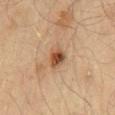  biopsy_status: not biopsied; imaged during a skin examination
  automated_metrics:
    border_irregularity_0_10: 2.5
    color_variation_0_10: 5.0
    peripheral_color_asymmetry: 1.5
    nevus_likeness_0_100: 95
  patient:
    sex: male
    age_approx: 45
  image:
    source: total-body photography crop
    field_of_view_mm: 15
  lighting: cross-polarized
  site: mid back
  lesion_size:
    long_diameter_mm_approx: 3.0